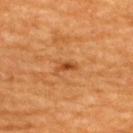| field | value |
|---|---|
| follow-up | imaged on a skin check; not biopsied |
| acquisition | 15 mm crop, total-body photography |
| size | ≈2.5 mm |
| subject | male, aged 58 to 62 |
| anatomic site | the back |